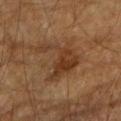| feature | finding |
|---|---|
| workup | no biopsy performed (imaged during a skin exam) |
| TBP lesion metrics | a footprint of about 14 mm², a shape eccentricity near 0.3, and two-axis asymmetry of about 0.7; an average lesion color of about L≈31 a*≈17 b*≈28 (CIELAB), a lesion–skin lightness drop of about 7, and a lesion-to-skin contrast of about 7.5 (normalized; higher = more distinct); a border-irregularity index near 9.5/10, a color-variation rating of about 3/10, and a peripheral color-asymmetry measure near 1; an automated nevus-likeness rating near 5 out of 100 and a lesion-detection confidence of about 100/100 |
| image source | ~15 mm tile from a whole-body skin photo |
| site | the right upper arm |
| lesion diameter | ~5.5 mm (longest diameter) |
| patient | male, aged 83 or older |
| illumination | cross-polarized |